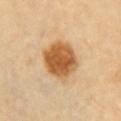Imaged during a routine full-body skin examination; the lesion was not biopsied and no histopathology is available. From the chest. The recorded lesion diameter is about 5 mm. A female patient, aged 58 to 62. A lesion tile, about 15 mm wide, cut from a 3D total-body photograph.